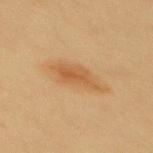Clinical impression: Captured during whole-body skin photography for melanoma surveillance; the lesion was not biopsied. Background: On the mid back. A female patient, approximately 20 years of age. Cropped from a total-body skin-imaging series; the visible field is about 15 mm. The tile uses cross-polarized illumination.